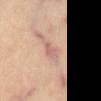follow-up: no biopsy performed (imaged during a skin exam) | body site: the front of the torso | size: ~2.5 mm (longest diameter) | TBP lesion metrics: a lesion area of about 3 mm² and an outline eccentricity of about 0.85 (0 = round, 1 = elongated); a detector confidence of about 60 out of 100 that the crop contains a lesion | image: 15 mm crop, total-body photography.